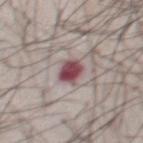The subject is a male in their mid- to late 30s.
Longest diameter approximately 3 mm.
This image is a 15 mm lesion crop taken from a total-body photograph.
The lesion is on the abdomen.
Captured under white-light illumination.